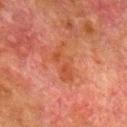Imaged during a routine full-body skin examination; the lesion was not biopsied and no histopathology is available. A 15 mm close-up extracted from a 3D total-body photography capture. The lesion is on the right lower leg. The total-body-photography lesion software estimated a symmetry-axis asymmetry near 0.45. The analysis additionally found border irregularity of about 6 on a 0–10 scale, internal color variation of about 3 on a 0–10 scale, and radial color variation of about 1. The software also gave a lesion-detection confidence of about 100/100. This is a cross-polarized tile. About 4 mm across. A male subject, aged 78–82.